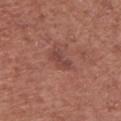notes: catalogued during a skin exam; not biopsied
image source: ~15 mm crop, total-body skin-cancer survey
body site: the chest
subject: male, in their mid- to late 70s